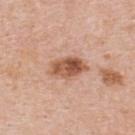The lesion was photographed on a routine skin check and not biopsied; there is no pathology result.
Located on the upper back.
Longest diameter approximately 4.5 mm.
The subject is a male in their 60s.
Cropped from a whole-body photographic skin survey; the tile spans about 15 mm.
This is a white-light tile.